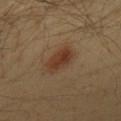| feature | finding |
|---|---|
| biopsy status | catalogued during a skin exam; not biopsied |
| body site | the chest |
| patient | male, approximately 40 years of age |
| lighting | cross-polarized illumination |
| image | ~15 mm crop, total-body skin-cancer survey |